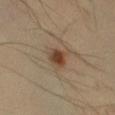| feature | finding |
|---|---|
| notes | total-body-photography surveillance lesion; no biopsy |
| body site | the right lower leg |
| image source | ~15 mm tile from a whole-body skin photo |
| subject | male, in their mid-40s |